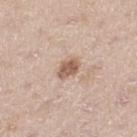The lesion was tiled from a total-body skin photograph and was not biopsied. Longest diameter approximately 3 mm. The lesion-visualizer software estimated border irregularity of about 2 on a 0–10 scale and peripheral color asymmetry of about 1. Located on the left thigh. This image is a 15 mm lesion crop taken from a total-body photograph. This is a white-light tile. The patient is a male approximately 65 years of age.